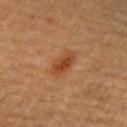The lesion was tiled from a total-body skin photograph and was not biopsied. The lesion is located on the right upper arm. Cropped from a whole-body photographic skin survey; the tile spans about 15 mm. A female subject roughly 60 years of age.